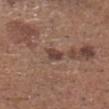Impression:
Part of a total-body skin-imaging series; this lesion was reviewed on a skin check and was not flagged for biopsy.
Background:
This image is a 15 mm lesion crop taken from a total-body photograph. Measured at roughly 2.5 mm in maximum diameter. A male subject aged 73–77. The tile uses white-light illumination. Automated image analysis of the tile measured an average lesion color of about L≈42 a*≈18 b*≈23 (CIELAB), roughly 10 lightness units darker than nearby skin, and a lesion-to-skin contrast of about 8.5 (normalized; higher = more distinct). And it measured a border-irregularity index near 3/10, a within-lesion color-variation index near 2/10, and peripheral color asymmetry of about 1. The software also gave a lesion-detection confidence of about 100/100. On the head or neck.